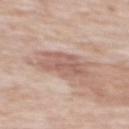Clinical impression: This lesion was catalogued during total-body skin photography and was not selected for biopsy. Clinical summary: The subject is a female about 75 years old. The lesion is located on the mid back. The lesion's longest dimension is about 4.5 mm. A lesion tile, about 15 mm wide, cut from a 3D total-body photograph.